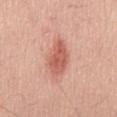The lesion was tiled from a total-body skin photograph and was not biopsied. Cropped from a whole-body photographic skin survey; the tile spans about 15 mm. The lesion-visualizer software estimated a footprint of about 10 mm², a shape eccentricity near 0.8, and a shape-asymmetry score of about 0.15 (0 = symmetric). The analysis additionally found a mean CIELAB color near L≈59 a*≈27 b*≈30, about 12 CIELAB-L* units darker than the surrounding skin, and a lesion-to-skin contrast of about 7.5 (normalized; higher = more distinct). The software also gave a border-irregularity index near 2/10, internal color variation of about 3.5 on a 0–10 scale, and radial color variation of about 1. Imaged with white-light lighting. On the back. The patient is a male in their 60s.